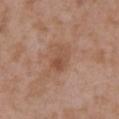Background:
The lesion is located on the right upper arm. The lesion-visualizer software estimated an area of roughly 6.5 mm², an eccentricity of roughly 0.8, and a shape-asymmetry score of about 0.25 (0 = symmetric). And it measured a lesion color around L≈51 a*≈21 b*≈30 in CIELAB, roughly 7 lightness units darker than nearby skin, and a normalized border contrast of about 6. The software also gave a color-variation rating of about 4.5/10. And it measured a nevus-likeness score of about 0/100 and lesion-presence confidence of about 100/100. This is a white-light tile. Measured at roughly 3.5 mm in maximum diameter. Cropped from a whole-body photographic skin survey; the tile spans about 15 mm. A female patient, aged 33 to 37.